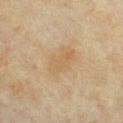The lesion was tiled from a total-body skin photograph and was not biopsied. Automated tile analysis of the lesion measured a mean CIELAB color near L≈50 a*≈12 b*≈30, a lesion–skin lightness drop of about 5, and a lesion-to-skin contrast of about 4.5 (normalized; higher = more distinct). And it measured a classifier nevus-likeness of about 0/100. A close-up tile cropped from a whole-body skin photograph, about 15 mm across. A male patient, approximately 70 years of age. The lesion is located on the front of the torso. This is a cross-polarized tile.